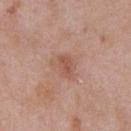Q: Was this lesion biopsied?
A: no biopsy performed (imaged during a skin exam)
Q: What is the anatomic site?
A: the chest
Q: Illumination type?
A: white-light
Q: What are the patient's age and sex?
A: male, approximately 55 years of age
Q: What kind of image is this?
A: ~15 mm tile from a whole-body skin photo
Q: How large is the lesion?
A: ≈2.5 mm